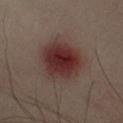workup: total-body-photography surveillance lesion; no biopsy | patient: roughly 55 years of age | image: 15 mm crop, total-body photography | site: the left upper arm.